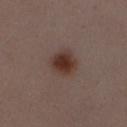Clinical impression:
Imaged during a routine full-body skin examination; the lesion was not biopsied and no histopathology is available.
Clinical summary:
A female subject aged approximately 30. Cropped from a whole-body photographic skin survey; the tile spans about 15 mm. Automated image analysis of the tile measured an area of roughly 8.5 mm² and an eccentricity of roughly 0.3. And it measured a lesion color around L≈35 a*≈17 b*≈22 in CIELAB, roughly 10 lightness units darker than nearby skin, and a lesion-to-skin contrast of about 10 (normalized; higher = more distinct). Captured under white-light illumination. Located on the mid back.